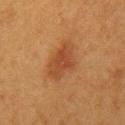Clinical impression: Captured during whole-body skin photography for melanoma surveillance; the lesion was not biopsied. Clinical summary: The lesion is located on the left upper arm. A female patient roughly 40 years of age. A close-up tile cropped from a whole-body skin photograph, about 15 mm across. The tile uses cross-polarized illumination.